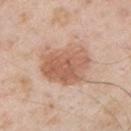Part of a total-body skin-imaging series; this lesion was reviewed on a skin check and was not flagged for biopsy.
Automated tile analysis of the lesion measured a lesion color around L≈60 a*≈21 b*≈31 in CIELAB, a lesion–skin lightness drop of about 12, and a normalized border contrast of about 8. It also reported a nevus-likeness score of about 95/100 and lesion-presence confidence of about 100/100.
Located on the left upper arm.
A male subject, roughly 55 years of age.
A 15 mm crop from a total-body photograph taken for skin-cancer surveillance.
Captured under white-light illumination.